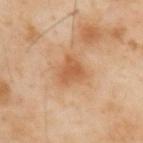Recorded during total-body skin imaging; not selected for excision or biopsy. Longest diameter approximately 3 mm. The patient is a male in their mid-50s. A 15 mm close-up tile from a total-body photography series done for melanoma screening. Located on the upper back. Imaged with cross-polarized lighting.